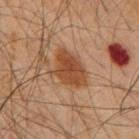The lesion was tiled from a total-body skin photograph and was not biopsied. This is a cross-polarized tile. A 15 mm crop from a total-body photograph taken for skin-cancer surveillance. Longest diameter approximately 5 mm. A male patient aged around 65. The total-body-photography lesion software estimated a nevus-likeness score of about 90/100 and a detector confidence of about 100 out of 100 that the crop contains a lesion. The lesion is on the mid back.